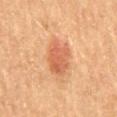Clinical impression:
No biopsy was performed on this lesion — it was imaged during a full skin examination and was not determined to be concerning.
Image and clinical context:
The lesion is located on the mid back. This is a cross-polarized tile. The recorded lesion diameter is about 4.5 mm. A 15 mm close-up extracted from a 3D total-body photography capture. A female subject, aged around 60.